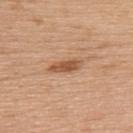{
  "biopsy_status": "not biopsied; imaged during a skin examination",
  "patient": {
    "sex": "male",
    "age_approx": 70
  },
  "site": "upper back",
  "image": {
    "source": "total-body photography crop",
    "field_of_view_mm": 15
  },
  "lesion_size": {
    "long_diameter_mm_approx": 4.0
  }
}